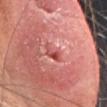The lesion was photographed on a routine skin check and not biopsied; there is no pathology result. This is a white-light tile. A 15 mm close-up extracted from a 3D total-body photography capture. The lesion is on the head or neck. About 2.5 mm across. The subject is a female approximately 55 years of age. An algorithmic analysis of the crop reported a lesion area of about 3 mm², an outline eccentricity of about 0.85 (0 = round, 1 = elongated), and a symmetry-axis asymmetry near 0.3. The software also gave a mean CIELAB color near L≈53 a*≈36 b*≈29, about 11 CIELAB-L* units darker than the surrounding skin, and a lesion-to-skin contrast of about 7.5 (normalized; higher = more distinct). The analysis additionally found a border-irregularity rating of about 3.5/10 and internal color variation of about 7.5 on a 0–10 scale. It also reported an automated nevus-likeness rating near 0 out of 100 and lesion-presence confidence of about 95/100.